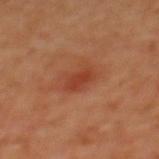Impression: The lesion was tiled from a total-body skin photograph and was not biopsied. Clinical summary: A female subject, about 40 years old. Cropped from a total-body skin-imaging series; the visible field is about 15 mm. An algorithmic analysis of the crop reported an area of roughly 4.5 mm². And it measured a mean CIELAB color near L≈42 a*≈30 b*≈34, about 8 CIELAB-L* units darker than the surrounding skin, and a lesion-to-skin contrast of about 6.5 (normalized; higher = more distinct). The analysis additionally found a classifier nevus-likeness of about 55/100. Captured under cross-polarized illumination. The lesion is located on the upper back.